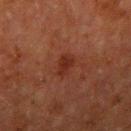The lesion was photographed on a routine skin check and not biopsied; there is no pathology result.
Cropped from a whole-body photographic skin survey; the tile spans about 15 mm.
A male subject, aged around 60.
The tile uses cross-polarized illumination.
From the right upper arm.